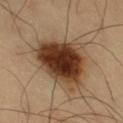Q: Was a biopsy performed?
A: no biopsy performed (imaged during a skin exam)
Q: What kind of image is this?
A: 15 mm crop, total-body photography
Q: Lesion location?
A: the right thigh
Q: How large is the lesion?
A: about 6.5 mm
Q: How was the tile lit?
A: cross-polarized
Q: Patient demographics?
A: male, about 60 years old